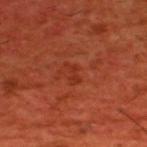{"biopsy_status": "not biopsied; imaged during a skin examination", "automated_metrics": {"area_mm2_approx": 2.5, "eccentricity": 0.9, "shape_asymmetry": 0.5, "cielab_L": 33, "cielab_a": 33, "cielab_b": 34, "vs_skin_darker_L": 5.0, "border_irregularity_0_10": 5.5, "color_variation_0_10": 0.0, "peripheral_color_asymmetry": 0.0, "nevus_likeness_0_100": 0}, "lighting": "cross-polarized", "patient": {"sex": "male", "age_approx": 60}, "site": "upper back", "image": {"source": "total-body photography crop", "field_of_view_mm": 15}}